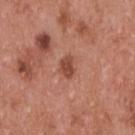• imaging modality: ~15 mm tile from a whole-body skin photo
• patient: male, aged 53 to 57
• body site: the upper back